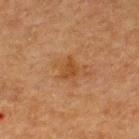No biopsy was performed on this lesion — it was imaged during a full skin examination and was not determined to be concerning.